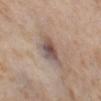Q: Was a biopsy performed?
A: total-body-photography surveillance lesion; no biopsy
Q: What lighting was used for the tile?
A: cross-polarized
Q: What is the anatomic site?
A: the left thigh
Q: How was this image acquired?
A: total-body-photography crop, ~15 mm field of view
Q: What are the patient's age and sex?
A: female, roughly 55 years of age
Q: What is the lesion's diameter?
A: ~3 mm (longest diameter)